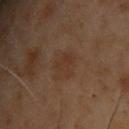Impression:
Recorded during total-body skin imaging; not selected for excision or biopsy.
Clinical summary:
A 15 mm close-up tile from a total-body photography series done for melanoma screening. The subject is a male about 55 years old. The tile uses cross-polarized illumination. Measured at roughly 3.5 mm in maximum diameter. On the chest.